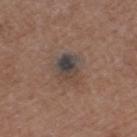Impression:
Part of a total-body skin-imaging series; this lesion was reviewed on a skin check and was not flagged for biopsy.
Acquisition and patient details:
The lesion-visualizer software estimated a mean CIELAB color near L≈40 a*≈10 b*≈18, about 10 CIELAB-L* units darker than the surrounding skin, and a normalized border contrast of about 10. The software also gave internal color variation of about 10 on a 0–10 scale and a peripheral color-asymmetry measure near 4. The subject is a male aged approximately 75. Imaged with white-light lighting. The lesion is located on the left thigh. A roughly 15 mm field-of-view crop from a total-body skin photograph. Longest diameter approximately 3.5 mm.